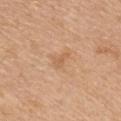notes: total-body-photography surveillance lesion; no biopsy | illumination: white-light illumination | subject: female, aged 53 to 57 | anatomic site: the upper back | acquisition: total-body-photography crop, ~15 mm field of view.